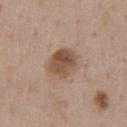Part of a total-body skin-imaging series; this lesion was reviewed on a skin check and was not flagged for biopsy. A male subject about 60 years old. The lesion is on the chest. About 4 mm across. A 15 mm close-up tile from a total-body photography series done for melanoma screening. Automated tile analysis of the lesion measured a shape eccentricity near 0.6 and two-axis asymmetry of about 0.15. The analysis additionally found an average lesion color of about L≈50 a*≈18 b*≈29 (CIELAB) and a lesion–skin lightness drop of about 12. It also reported a border-irregularity rating of about 1.5/10, a within-lesion color-variation index near 5/10, and a peripheral color-asymmetry measure near 2. It also reported a classifier nevus-likeness of about 75/100.